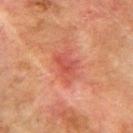Clinical impression:
This lesion was catalogued during total-body skin photography and was not selected for biopsy.
Background:
A lesion tile, about 15 mm wide, cut from a 3D total-body photograph. A male patient, aged approximately 75. Automated tile analysis of the lesion measured a footprint of about 6.5 mm², an outline eccentricity of about 0.75 (0 = round, 1 = elongated), and a shape-asymmetry score of about 0.35 (0 = symmetric). The software also gave a mean CIELAB color near L≈44 a*≈30 b*≈28, a lesion–skin lightness drop of about 8, and a normalized border contrast of about 6. The software also gave a within-lesion color-variation index near 3/10 and peripheral color asymmetry of about 1. About 3.5 mm across. From the right upper arm.